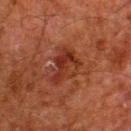Q: Is there a histopathology result?
A: total-body-photography surveillance lesion; no biopsy
Q: Where on the body is the lesion?
A: the upper back
Q: What kind of image is this?
A: ~15 mm tile from a whole-body skin photo
Q: What are the patient's age and sex?
A: male, aged approximately 60